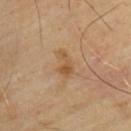Notes:
• follow-up — catalogued during a skin exam; not biopsied
• lighting — cross-polarized illumination
• acquisition — ~15 mm crop, total-body skin-cancer survey
• image-analysis metrics — border irregularity of about 4.5 on a 0–10 scale, a within-lesion color-variation index near 2.5/10, and a peripheral color-asymmetry measure near 0.5; a classifier nevus-likeness of about 0/100 and a lesion-detection confidence of about 100/100
• subject — male, aged 63 to 67
• lesion size — ~3.5 mm (longest diameter)
• site — the upper back